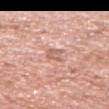Clinical impression:
The lesion was photographed on a routine skin check and not biopsied; there is no pathology result.
Background:
A male subject, about 70 years old. Automated tile analysis of the lesion measured an average lesion color of about L≈62 a*≈23 b*≈29 (CIELAB), about 9 CIELAB-L* units darker than the surrounding skin, and a normalized border contrast of about 6. The software also gave a border-irregularity index near 2.5/10, internal color variation of about 1.5 on a 0–10 scale, and peripheral color asymmetry of about 0.5. The software also gave a classifier nevus-likeness of about 0/100 and lesion-presence confidence of about 95/100. A 15 mm close-up tile from a total-body photography series done for melanoma screening. About 2.5 mm across. The tile uses white-light illumination. From the right upper arm.